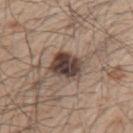The lesion was photographed on a routine skin check and not biopsied; there is no pathology result. From the arm. About 4 mm across. The subject is a male aged approximately 50. A roughly 15 mm field-of-view crop from a total-body skin photograph.